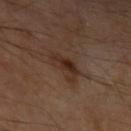notes: imaged on a skin check; not biopsied
imaging modality: ~15 mm crop, total-body skin-cancer survey
body site: the arm
subject: male, aged 63–67
lesion diameter: ≈3.5 mm